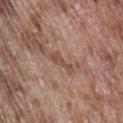Assessment: Imaged during a routine full-body skin examination; the lesion was not biopsied and no histopathology is available. Context: Automated tile analysis of the lesion measured a shape-asymmetry score of about 0.5 (0 = symmetric). The analysis additionally found a lesion color around L≈49 a*≈19 b*≈27 in CIELAB, roughly 8 lightness units darker than nearby skin, and a normalized lesion–skin contrast near 6.5. The analysis additionally found a border-irregularity index near 6/10, a color-variation rating of about 0/10, and radial color variation of about 0. A region of skin cropped from a whole-body photographic capture, roughly 15 mm wide. This is a white-light tile. On the abdomen. A male subject, in their mid- to late 70s. About 3.5 mm across.